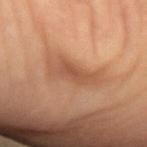follow-up = total-body-photography surveillance lesion; no biopsy | patient = female, approximately 50 years of age | diameter = ≈6 mm | image-analysis metrics = an area of roughly 9.5 mm² and a symmetry-axis asymmetry near 0.35; border irregularity of about 4.5 on a 0–10 scale, internal color variation of about 3 on a 0–10 scale, and radial color variation of about 1 | acquisition = ~15 mm tile from a whole-body skin photo | tile lighting = cross-polarized | location = the left forearm.